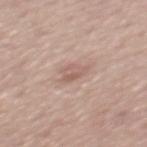<record>
  <biopsy_status>not biopsied; imaged during a skin examination</biopsy_status>
  <lighting>white-light</lighting>
  <patient>
    <sex>male</sex>
    <age_approx>50</age_approx>
  </patient>
  <automated_metrics>
    <border_irregularity_0_10>3.5</border_irregularity_0_10>
    <color_variation_0_10>4.0</color_variation_0_10>
    <peripheral_color_asymmetry>1.5</peripheral_color_asymmetry>
    <nevus_likeness_0_100>5</nevus_likeness_0_100>
  </automated_metrics>
  <lesion_size>
    <long_diameter_mm_approx>3.0</long_diameter_mm_approx>
  </lesion_size>
  <image>
    <source>total-body photography crop</source>
    <field_of_view_mm>15</field_of_view_mm>
  </image>
  <site>mid back</site>
</record>